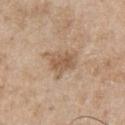<case>
  <biopsy_status>not biopsied; imaged during a skin examination</biopsy_status>
  <image>
    <source>total-body photography crop</source>
    <field_of_view_mm>15</field_of_view_mm>
  </image>
  <site>chest</site>
  <lighting>white-light</lighting>
  <lesion_size>
    <long_diameter_mm_approx>4.0</long_diameter_mm_approx>
  </lesion_size>
  <patient>
    <sex>male</sex>
    <age_approx>65</age_approx>
  </patient>
</case>